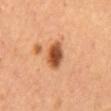Findings:
– biopsy status — total-body-photography surveillance lesion; no biopsy
– automated lesion analysis — a footprint of about 7 mm², a shape eccentricity near 0.75, and two-axis asymmetry of about 0.15
– location — the abdomen
– patient — female
– lighting — cross-polarized illumination
– acquisition — ~15 mm crop, total-body skin-cancer survey